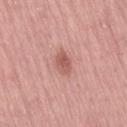Clinical impression: The lesion was photographed on a routine skin check and not biopsied; there is no pathology result. Image and clinical context: Automated image analysis of the tile measured a footprint of about 4.5 mm², a shape eccentricity near 0.75, and two-axis asymmetry of about 0.25. The analysis additionally found roughly 10 lightness units darker than nearby skin and a lesion-to-skin contrast of about 7 (normalized; higher = more distinct). And it measured a classifier nevus-likeness of about 40/100. The patient is a male in their mid- to late 50s. Captured under white-light illumination. Cropped from a whole-body photographic skin survey; the tile spans about 15 mm. The lesion is located on the right thigh. Longest diameter approximately 3 mm.